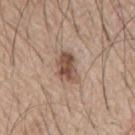Findings:
* workup · total-body-photography surveillance lesion; no biopsy
* tile lighting · white-light illumination
* diameter · ≈4 mm
* subject · male, aged around 65
* image source · 15 mm crop, total-body photography
* anatomic site · the mid back